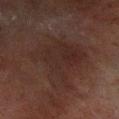Clinical impression:
Recorded during total-body skin imaging; not selected for excision or biopsy.
Clinical summary:
Located on the left lower leg. A male patient, approximately 70 years of age. Measured at roughly 8 mm in maximum diameter. Captured under cross-polarized illumination. The total-body-photography lesion software estimated a border-irregularity index near 5.5/10, a within-lesion color-variation index near 3.5/10, and a peripheral color-asymmetry measure near 1. It also reported a nevus-likeness score of about 0/100 and a lesion-detection confidence of about 100/100. This image is a 15 mm lesion crop taken from a total-body photograph.